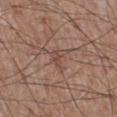• notes — imaged on a skin check; not biopsied
• location — the right lower leg
• lighting — white-light
• image — ~15 mm tile from a whole-body skin photo
• patient — male, in their mid- to late 60s
• lesion size — ~3 mm (longest diameter)